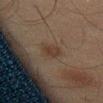| feature | finding |
|---|---|
| workup | no biopsy performed (imaged during a skin exam) |
| illumination | cross-polarized |
| diameter | about 3 mm |
| anatomic site | the left thigh |
| patient | male, aged 43–47 |
| automated lesion analysis | a lesion area of about 5 mm², a shape eccentricity near 0.65, and a shape-asymmetry score of about 0.2 (0 = symmetric); roughly 5 lightness units darker than nearby skin and a normalized lesion–skin contrast near 6.5; a detector confidence of about 100 out of 100 that the crop contains a lesion |
| acquisition | ~15 mm crop, total-body skin-cancer survey |